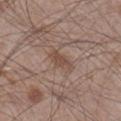{
  "biopsy_status": "not biopsied; imaged during a skin examination",
  "patient": {
    "sex": "male",
    "age_approx": 45
  },
  "lesion_size": {
    "long_diameter_mm_approx": 3.0
  },
  "image": {
    "source": "total-body photography crop",
    "field_of_view_mm": 15
  },
  "automated_metrics": {
    "border_irregularity_0_10": 4.5,
    "peripheral_color_asymmetry": 0.5
  },
  "site": "right lower leg",
  "lighting": "white-light"
}